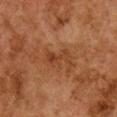| field | value |
|---|---|
| follow-up | catalogued during a skin exam; not biopsied |
| size | ≈4 mm |
| body site | the chest |
| TBP lesion metrics | an eccentricity of roughly 0.9 and a symmetry-axis asymmetry near 0.4; a within-lesion color-variation index near 4/10 |
| image source | ~15 mm crop, total-body skin-cancer survey |
| lighting | cross-polarized illumination |
| subject | female, roughly 60 years of age |